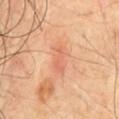Clinical impression:
Captured during whole-body skin photography for melanoma surveillance; the lesion was not biopsied.
Acquisition and patient details:
An algorithmic analysis of the crop reported a mean CIELAB color near L≈65 a*≈28 b*≈36 and a lesion-to-skin contrast of about 4.5 (normalized; higher = more distinct). The analysis additionally found border irregularity of about 3.5 on a 0–10 scale and a peripheral color-asymmetry measure near 0.5. And it measured a classifier nevus-likeness of about 0/100 and a lesion-detection confidence of about 100/100. The lesion's longest dimension is about 4 mm. A male patient, aged around 65. This image is a 15 mm lesion crop taken from a total-body photograph. Located on the front of the torso.